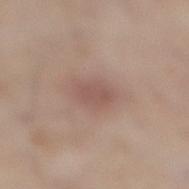Impression: The lesion was photographed on a routine skin check and not biopsied; there is no pathology result. Acquisition and patient details: An algorithmic analysis of the crop reported a lesion area of about 5.5 mm² and two-axis asymmetry of about 0.15. The analysis additionally found an automated nevus-likeness rating near 10 out of 100. A 15 mm crop from a total-body photograph taken for skin-cancer surveillance. A male subject, in their 60s. Located on the leg. Captured under white-light illumination. About 3.5 mm across.